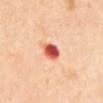workup = total-body-photography surveillance lesion; no biopsy
automated metrics = a footprint of about 5.5 mm², an eccentricity of roughly 0.35, and a symmetry-axis asymmetry near 0.15; a mean CIELAB color near L≈61 a*≈39 b*≈35, about 20 CIELAB-L* units darker than the surrounding skin, and a normalized border contrast of about 11.5; a color-variation rating of about 7.5/10 and a peripheral color-asymmetry measure near 2
acquisition = ~15 mm crop, total-body skin-cancer survey
tile lighting = cross-polarized
diameter = ~2.5 mm (longest diameter)
subject = female, aged approximately 55
location = the abdomen